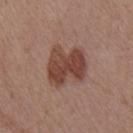Notes:
• follow-up: total-body-photography surveillance lesion; no biopsy
• subject: male, approximately 55 years of age
• image: ~15 mm crop, total-body skin-cancer survey
• location: the front of the torso